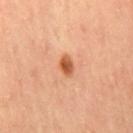acquisition: ~15 mm tile from a whole-body skin photo | illumination: cross-polarized illumination | size: ~2.5 mm (longest diameter) | subject: female, aged 58–62 | anatomic site: the left leg | automated metrics: an area of roughly 3.5 mm², an eccentricity of roughly 0.75, and a shape-asymmetry score of about 0.3 (0 = symmetric); a classifier nevus-likeness of about 100/100 and a detector confidence of about 100 out of 100 that the crop contains a lesion.